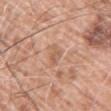• notes · catalogued during a skin exam; not biopsied
• location · the right upper arm
• diameter · about 3 mm
• imaging modality · total-body-photography crop, ~15 mm field of view
• patient · male, about 60 years old
• automated metrics · a mean CIELAB color near L≈60 a*≈21 b*≈31; border irregularity of about 3 on a 0–10 scale
• illumination · white-light